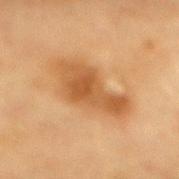Q: Is there a histopathology result?
A: total-body-photography surveillance lesion; no biopsy
Q: What kind of image is this?
A: ~15 mm tile from a whole-body skin photo
Q: Who is the patient?
A: female, aged 78 to 82
Q: Lesion location?
A: the mid back
Q: Illumination type?
A: cross-polarized illumination